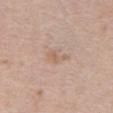The lesion was tiled from a total-body skin photograph and was not biopsied. Located on the abdomen. Imaged with white-light lighting. A male patient aged approximately 80. An algorithmic analysis of the crop reported a lesion area of about 3.5 mm² and a shape eccentricity near 0.8. And it measured an automated nevus-likeness rating near 0 out of 100 and lesion-presence confidence of about 100/100. A roughly 15 mm field-of-view crop from a total-body skin photograph.